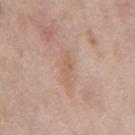The lesion was photographed on a routine skin check and not biopsied; there is no pathology result.
This is a white-light tile.
A male subject, aged approximately 60.
The total-body-photography lesion software estimated a lesion color around L≈60 a*≈18 b*≈30 in CIELAB, a lesion–skin lightness drop of about 6, and a normalized border contrast of about 5. The analysis additionally found an automated nevus-likeness rating near 0 out of 100 and lesion-presence confidence of about 100/100.
A close-up tile cropped from a whole-body skin photograph, about 15 mm across.
Approximately 4 mm at its widest.
The lesion is located on the mid back.